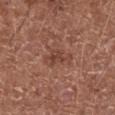<lesion>
<biopsy_status>not biopsied; imaged during a skin examination</biopsy_status>
<image>
  <source>total-body photography crop</source>
  <field_of_view_mm>15</field_of_view_mm>
</image>
<patient>
  <sex>male</sex>
  <age_approx>65</age_approx>
</patient>
<lesion_size>
  <long_diameter_mm_approx>3.5</long_diameter_mm_approx>
</lesion_size>
<automated_metrics>
  <area_mm2_approx>5.5</area_mm2_approx>
  <eccentricity>0.8</eccentricity>
</automated_metrics>
<site>abdomen</site>
<lighting>white-light</lighting>
</lesion>